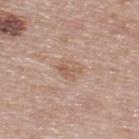– follow-up: imaged on a skin check; not biopsied
– site: the upper back
– imaging modality: 15 mm crop, total-body photography
– subject: male, about 55 years old